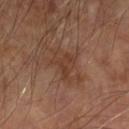notes = imaged on a skin check; not biopsied | anatomic site = the left forearm | imaging modality = ~15 mm tile from a whole-body skin photo | patient = male, in their 70s | automated metrics = a border-irregularity rating of about 6/10, a color-variation rating of about 2.5/10, and a peripheral color-asymmetry measure near 1 | size = ~3.5 mm (longest diameter).